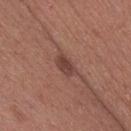Assessment:
This lesion was catalogued during total-body skin photography and was not selected for biopsy.
Image and clinical context:
The lesion is on the right thigh. Measured at roughly 2.5 mm in maximum diameter. The subject is a female aged approximately 40. A 15 mm crop from a total-body photograph taken for skin-cancer surveillance. This is a white-light tile.